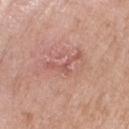| feature | finding |
|---|---|
| biopsy status | total-body-photography surveillance lesion; no biopsy |
| site | the right forearm |
| automated lesion analysis | a footprint of about 4 mm² and a symmetry-axis asymmetry near 0.85; a lesion color around L≈56 a*≈26 b*≈26 in CIELAB and roughly 8 lightness units darker than nearby skin; a border-irregularity rating of about 10/10, a color-variation rating of about 0/10, and a peripheral color-asymmetry measure near 0; a classifier nevus-likeness of about 0/100 and lesion-presence confidence of about 95/100 |
| diameter | ~4.5 mm (longest diameter) |
| imaging modality | ~15 mm crop, total-body skin-cancer survey |
| lighting | white-light illumination |
| subject | female, about 65 years old |